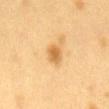{
  "site": "back",
  "image": {
    "source": "total-body photography crop",
    "field_of_view_mm": 15
  },
  "lighting": "cross-polarized",
  "lesion_size": {
    "long_diameter_mm_approx": 2.5
  },
  "automated_metrics": {
    "area_mm2_approx": 4.5,
    "eccentricity": 0.7,
    "shape_asymmetry": 0.25,
    "border_irregularity_0_10": 2.0
  },
  "patient": {
    "sex": "female",
    "age_approx": 40
  }
}